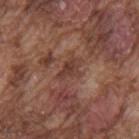Notes:
* diameter: about 3 mm
* acquisition: ~15 mm tile from a whole-body skin photo
* site: the upper back
* patient: male, aged approximately 75
* illumination: white-light